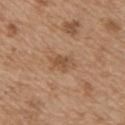No biopsy was performed on this lesion — it was imaged during a full skin examination and was not determined to be concerning. The tile uses white-light illumination. The lesion is on the chest. This image is a 15 mm lesion crop taken from a total-body photograph. The total-body-photography lesion software estimated a lesion area of about 4.5 mm², a shape eccentricity near 0.8, and a symmetry-axis asymmetry near 0.3. And it measured a within-lesion color-variation index near 2.5/10 and a peripheral color-asymmetry measure near 0.5. The subject is a male aged approximately 50.